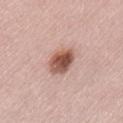The lesion was photographed on a routine skin check and not biopsied; there is no pathology result. A 15 mm close-up tile from a total-body photography series done for melanoma screening. Located on the lower back. The lesion-visualizer software estimated a lesion color around L≈53 a*≈23 b*≈27 in CIELAB and a normalized border contrast of about 11. The analysis additionally found a border-irregularity index near 2/10, a within-lesion color-variation index near 6.5/10, and radial color variation of about 2.5. A female subject aged 68 to 72.